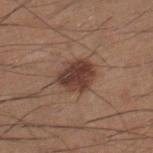A male patient aged 33 to 37. Captured under white-light illumination. A roughly 15 mm field-of-view crop from a total-body skin photograph. The total-body-photography lesion software estimated a footprint of about 11 mm² and a shape-asymmetry score of about 0.2 (0 = symmetric). The analysis additionally found a border-irregularity index near 2/10, a within-lesion color-variation index near 3.5/10, and a peripheral color-asymmetry measure near 1. And it measured a nevus-likeness score of about 85/100 and a detector confidence of about 100 out of 100 that the crop contains a lesion. The lesion's longest dimension is about 4 mm. From the right lower leg.